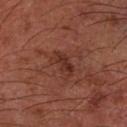Captured during whole-body skin photography for melanoma surveillance; the lesion was not biopsied. An algorithmic analysis of the crop reported a mean CIELAB color near L≈31 a*≈23 b*≈25 and a normalized lesion–skin contrast near 6.5. The analysis additionally found a border-irregularity rating of about 3.5/10, a color-variation rating of about 2.5/10, and radial color variation of about 1. Imaged with cross-polarized lighting. The lesion's longest dimension is about 3.5 mm. The lesion is on the left forearm. A roughly 15 mm field-of-view crop from a total-body skin photograph. A male subject in their 60s.